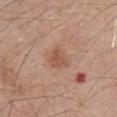Captured during whole-body skin photography for melanoma surveillance; the lesion was not biopsied.
The lesion is located on the abdomen.
A male subject, about 80 years old.
Imaged with white-light lighting.
A 15 mm close-up extracted from a 3D total-body photography capture.
Longest diameter approximately 3 mm.
Automated image analysis of the tile measured a border-irregularity index near 2/10, internal color variation of about 2.5 on a 0–10 scale, and peripheral color asymmetry of about 1. It also reported a nevus-likeness score of about 0/100 and a detector confidence of about 100 out of 100 that the crop contains a lesion.